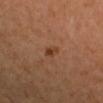{"biopsy_status": "not biopsied; imaged during a skin examination", "image": {"source": "total-body photography crop", "field_of_view_mm": 15}, "site": "right forearm", "patient": {"sex": "female", "age_approx": 30}, "lesion_size": {"long_diameter_mm_approx": 2.0}, "lighting": "cross-polarized"}